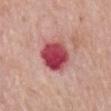The lesion was photographed on a routine skin check and not biopsied; there is no pathology result.
The subject is a male about 75 years old.
The lesion is located on the mid back.
This image is a 15 mm lesion crop taken from a total-body photograph.